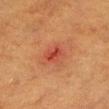Impression: This lesion was catalogued during total-body skin photography and was not selected for biopsy. Clinical summary: Imaged with cross-polarized lighting. Automated tile analysis of the lesion measured an area of roughly 3.5 mm², an outline eccentricity of about 0.8 (0 = round, 1 = elongated), and two-axis asymmetry of about 0.3. The software also gave a mean CIELAB color near L≈41 a*≈33 b*≈33 and a lesion–skin lightness drop of about 8. The analysis additionally found border irregularity of about 3 on a 0–10 scale and internal color variation of about 2.5 on a 0–10 scale. Measured at roughly 2.5 mm in maximum diameter. The lesion is located on the head or neck. A 15 mm crop from a total-body photograph taken for skin-cancer surveillance. A male subject, in their mid- to late 80s or older.